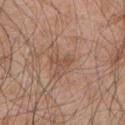{
  "biopsy_status": "not biopsied; imaged during a skin examination",
  "lesion_size": {
    "long_diameter_mm_approx": 2.5
  },
  "image": {
    "source": "total-body photography crop",
    "field_of_view_mm": 15
  },
  "site": "left upper arm",
  "patient": {
    "sex": "male",
    "age_approx": 45
  }
}